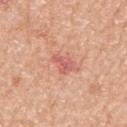| field | value |
|---|---|
| biopsy status | total-body-photography surveillance lesion; no biopsy |
| diameter | ~2.5 mm (longest diameter) |
| anatomic site | the left upper arm |
| acquisition | total-body-photography crop, ~15 mm field of view |
| tile lighting | white-light |
| patient | female, aged 73 to 77 |